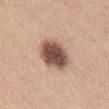Findings:
- diameter · ≈4.5 mm
- anatomic site · the lower back
- image-analysis metrics · an area of roughly 14 mm², a shape eccentricity near 0.6, and a symmetry-axis asymmetry near 0.15
- illumination · white-light illumination
- imaging modality · ~15 mm crop, total-body skin-cancer survey
- patient · female, aged around 25